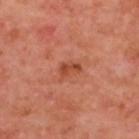Clinical impression:
Imaged during a routine full-body skin examination; the lesion was not biopsied and no histopathology is available.
Context:
Automated tile analysis of the lesion measured an average lesion color of about L≈47 a*≈29 b*≈35 (CIELAB), a lesion–skin lightness drop of about 9, and a normalized border contrast of about 7. It also reported a nevus-likeness score of about 50/100. The lesion's longest dimension is about 2.5 mm. A male patient in their 50s. A lesion tile, about 15 mm wide, cut from a 3D total-body photograph. This is a cross-polarized tile. From the upper back.